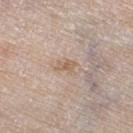* follow-up · catalogued during a skin exam; not biopsied
* automated lesion analysis · a border-irregularity rating of about 4/10, a within-lesion color-variation index near 1/10, and radial color variation of about 0
* image source · 15 mm crop, total-body photography
* tile lighting · white-light
* size · ≈2.5 mm
* patient · male, aged around 80
* body site · the right thigh